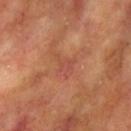workup: imaged on a skin check; not biopsied
imaging modality: 15 mm crop, total-body photography
body site: the right upper arm
automated lesion analysis: a lesion area of about 4 mm², an outline eccentricity of about 0.8 (0 = round, 1 = elongated), and a symmetry-axis asymmetry near 0.5; roughly 6 lightness units darker than nearby skin; internal color variation of about 1.5 on a 0–10 scale; a classifier nevus-likeness of about 0/100
lesion size: ~3 mm (longest diameter)
subject: female, roughly 75 years of age